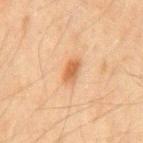Assessment:
Part of a total-body skin-imaging series; this lesion was reviewed on a skin check and was not flagged for biopsy.
Clinical summary:
A male patient, in their mid- to late 40s. Located on the mid back. This image is a 15 mm lesion crop taken from a total-body photograph. This is a cross-polarized tile.